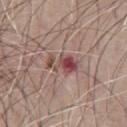Clinical impression: The lesion was tiled from a total-body skin photograph and was not biopsied. Context: The recorded lesion diameter is about 4 mm. A male patient, approximately 65 years of age. On the chest. Captured under white-light illumination. Cropped from a whole-body photographic skin survey; the tile spans about 15 mm. The total-body-photography lesion software estimated an average lesion color of about L≈47 a*≈23 b*≈21 (CIELAB), roughly 12 lightness units darker than nearby skin, and a normalized border contrast of about 9. The analysis additionally found a border-irregularity rating of about 6.5/10, a within-lesion color-variation index near 4.5/10, and radial color variation of about 1.